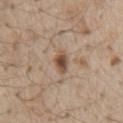  biopsy_status: not biopsied; imaged during a skin examination
  image:
    source: total-body photography crop
    field_of_view_mm: 15
  patient:
    sex: male
    age_approx: 70
  site: chest